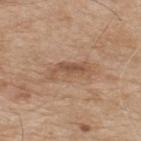No biopsy was performed on this lesion — it was imaged during a full skin examination and was not determined to be concerning. On the upper back. A male subject, aged around 75. The total-body-photography lesion software estimated a within-lesion color-variation index near 2/10 and radial color variation of about 0.5. A 15 mm crop from a total-body photograph taken for skin-cancer surveillance. This is a white-light tile. Approximately 5 mm at its widest.